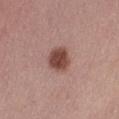Findings:
* follow-up — imaged on a skin check; not biopsied
* diameter — about 3 mm
* patient — female, about 55 years old
* location — the left thigh
* imaging modality — ~15 mm tile from a whole-body skin photo